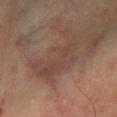Assessment: Recorded during total-body skin imaging; not selected for excision or biopsy. Acquisition and patient details: On the left lower leg. The tile uses cross-polarized illumination. A 15 mm close-up extracted from a 3D total-body photography capture. Approximately 7 mm at its widest. The patient is a male approximately 45 years of age.